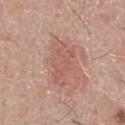Part of a total-body skin-imaging series; this lesion was reviewed on a skin check and was not flagged for biopsy. The lesion is on the front of the torso. Captured under white-light illumination. A lesion tile, about 15 mm wide, cut from a 3D total-body photograph. A male subject in their mid-40s. Longest diameter approximately 5.5 mm. Automated image analysis of the tile measured a footprint of about 12 mm², a shape eccentricity near 0.85, and a shape-asymmetry score of about 0.35 (0 = symmetric).